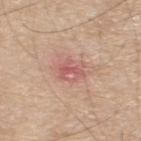Image and clinical context: A 15 mm crop from a total-body photograph taken for skin-cancer surveillance. A male patient, in their 70s. From the upper back. The tile uses white-light illumination. About 3.5 mm across.